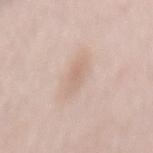{"biopsy_status": "not biopsied; imaged during a skin examination", "lesion_size": {"long_diameter_mm_approx": 4.0}, "lighting": "white-light", "patient": {"sex": "female", "age_approx": 55}, "site": "mid back", "image": {"source": "total-body photography crop", "field_of_view_mm": 15}}